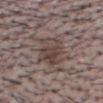No biopsy was performed on this lesion — it was imaged during a full skin examination and was not determined to be concerning.
On the head or neck.
The patient is a male about 40 years old.
Captured under white-light illumination.
A 15 mm close-up tile from a total-body photography series done for melanoma screening.
Approximately 4.5 mm at its widest.